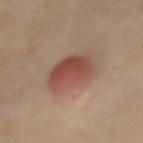Q: Was this lesion biopsied?
A: catalogued during a skin exam; not biopsied
Q: Lesion location?
A: the chest
Q: What is the imaging modality?
A: 15 mm crop, total-body photography